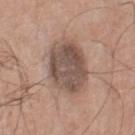The lesion was photographed on a routine skin check and not biopsied; there is no pathology result. Located on the right thigh. A roughly 15 mm field-of-view crop from a total-body skin photograph. A male subject aged 73–77. Longest diameter approximately 6.5 mm. Imaged with white-light lighting.